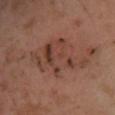On the leg. A 15 mm close-up tile from a total-body photography series done for melanoma screening. Imaged with cross-polarized lighting. A male patient, aged approximately 30. Measured at roughly 8 mm in maximum diameter.